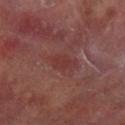Case summary:
• notes · no biopsy performed (imaged during a skin exam)
• image source · 15 mm crop, total-body photography
• diameter · about 3 mm
• patient · male, aged approximately 70
• site · the leg
• tile lighting · cross-polarized illumination